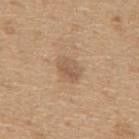Impression:
No biopsy was performed on this lesion — it was imaged during a full skin examination and was not determined to be concerning.
Background:
This image is a 15 mm lesion crop taken from a total-body photograph. The lesion's longest dimension is about 3 mm. Located on the mid back. The total-body-photography lesion software estimated a border-irregularity rating of about 2/10. And it measured lesion-presence confidence of about 100/100. Captured under white-light illumination. A male patient approximately 65 years of age.